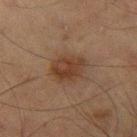notes: no biopsy performed (imaged during a skin exam); image source: 15 mm crop, total-body photography; subject: male, in their mid-60s; lesion size: about 4 mm; site: the left thigh.